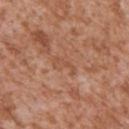Q: Is there a histopathology result?
A: total-body-photography surveillance lesion; no biopsy
Q: Lesion size?
A: ~3 mm (longest diameter)
Q: What is the imaging modality?
A: ~15 mm tile from a whole-body skin photo
Q: Illumination type?
A: white-light illumination
Q: What is the anatomic site?
A: the arm
Q: Patient demographics?
A: male, aged 43 to 47